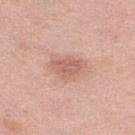Recorded during total-body skin imaging; not selected for excision or biopsy.
Approximately 4 mm at its widest.
A 15 mm close-up tile from a total-body photography series done for melanoma screening.
A female subject approximately 40 years of age.
The lesion-visualizer software estimated a lesion–skin lightness drop of about 10 and a normalized lesion–skin contrast near 6. And it measured a border-irregularity rating of about 2.5/10 and a color-variation rating of about 2.5/10.
The lesion is on the right lower leg.
The tile uses white-light illumination.